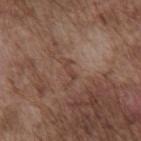Impression:
Part of a total-body skin-imaging series; this lesion was reviewed on a skin check and was not flagged for biopsy.
Acquisition and patient details:
Located on the chest. A male subject aged 73–77. This image is a 15 mm lesion crop taken from a total-body photograph.